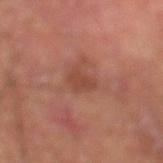| key | value |
|---|---|
| follow-up | total-body-photography surveillance lesion; no biopsy |
| subject | male, in their 70s |
| body site | the left lower leg |
| image source | 15 mm crop, total-body photography |